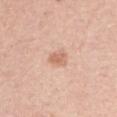Q: Is there a histopathology result?
A: catalogued during a skin exam; not biopsied
Q: What are the patient's age and sex?
A: male, in their mid-40s
Q: How was the tile lit?
A: white-light
Q: How was this image acquired?
A: ~15 mm crop, total-body skin-cancer survey
Q: What is the lesion's diameter?
A: ~2.5 mm (longest diameter)
Q: Lesion location?
A: the arm